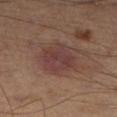| field | value |
|---|---|
| notes | imaged on a skin check; not biopsied |
| site | the leg |
| image | total-body-photography crop, ~15 mm field of view |
| illumination | cross-polarized |
| TBP lesion metrics | a lesion area of about 13 mm², an eccentricity of roughly 0.45, and two-axis asymmetry of about 0.15; about 7 CIELAB-L* units darker than the surrounding skin and a normalized border contrast of about 7; a border-irregularity rating of about 1.5/10, internal color variation of about 3.5 on a 0–10 scale, and radial color variation of about 1 |
| lesion diameter | ≈4.5 mm |
| subject | male, in their 50s |